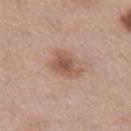| feature | finding |
|---|---|
| workup | catalogued during a skin exam; not biopsied |
| subject | female, aged approximately 40 |
| TBP lesion metrics | a within-lesion color-variation index near 3/10 |
| image | ~15 mm crop, total-body skin-cancer survey |
| body site | the left thigh |
| diameter | about 3.5 mm |
| illumination | white-light illumination |